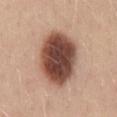This is a white-light tile.
The lesion is located on the mid back.
A female subject about 45 years old.
Automated tile analysis of the lesion measured an area of roughly 28 mm². The software also gave an automated nevus-likeness rating near 75 out of 100 and a detector confidence of about 100 out of 100 that the crop contains a lesion.
Cropped from a whole-body photographic skin survey; the tile spans about 15 mm.
Approximately 7.5 mm at its widest.